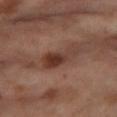Captured during whole-body skin photography for melanoma surveillance; the lesion was not biopsied. The subject is a female aged around 55. A 15 mm close-up tile from a total-body photography series done for melanoma screening. From the right thigh.